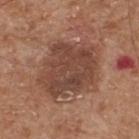The lesion was tiled from a total-body skin photograph and was not biopsied. A 15 mm crop from a total-body photograph taken for skin-cancer surveillance. A male patient, in their mid-60s. From the upper back. Longest diameter approximately 7 mm.